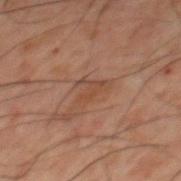Assessment: The lesion was photographed on a routine skin check and not biopsied; there is no pathology result. Image and clinical context: A roughly 15 mm field-of-view crop from a total-body skin photograph. The patient is a male about 60 years old. The lesion is located on the mid back.